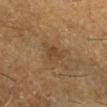Notes:
* biopsy status — no biopsy performed (imaged during a skin exam)
* automated metrics — lesion-presence confidence of about 100/100
* lighting — cross-polarized illumination
* patient — male, approximately 60 years of age
* acquisition — 15 mm crop, total-body photography
* anatomic site — the right lower leg
* lesion size — ~2.5 mm (longest diameter)